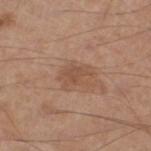  biopsy_status: not biopsied; imaged during a skin examination
  patient:
    sex: male
    age_approx: 55
  lesion_size:
    long_diameter_mm_approx: 4.0
  automated_metrics:
    eccentricity: 0.75
    shape_asymmetry: 0.3
    cielab_L: 51
    cielab_a: 20
    cielab_b: 30
    vs_skin_darker_L: 8.0
    vs_skin_contrast_norm: 5.5
    nevus_likeness_0_100: 5
    lesion_detection_confidence_0_100: 100
  image:
    source: total-body photography crop
    field_of_view_mm: 15
  site: left lower leg